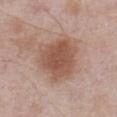notes: total-body-photography surveillance lesion; no biopsy | patient: male, in their mid-50s | site: the abdomen | image: ~15 mm tile from a whole-body skin photo.